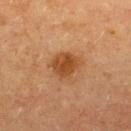A female patient aged approximately 55.
Cropped from a total-body skin-imaging series; the visible field is about 15 mm.
The lesion is located on the upper back.
The lesion-visualizer software estimated a footprint of about 9 mm² and an eccentricity of roughly 0.45. The software also gave a nevus-likeness score of about 95/100 and a lesion-detection confidence of about 100/100.